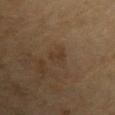Findings:
• biopsy status: imaged on a skin check; not biopsied
• tile lighting: cross-polarized illumination
• subject: male, aged 68–72
• automated metrics: a border-irregularity index near 3.5/10 and radial color variation of about 0.5; a classifier nevus-likeness of about 5/100 and a lesion-detection confidence of about 100/100
• lesion diameter: ≈2.5 mm
• acquisition: 15 mm crop, total-body photography
• anatomic site: the left upper arm